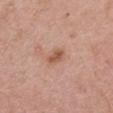Captured during whole-body skin photography for melanoma surveillance; the lesion was not biopsied. This image is a 15 mm lesion crop taken from a total-body photograph. The subject is a female about 45 years old. From the right upper arm.